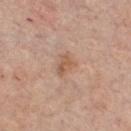| feature | finding |
|---|---|
| workup | catalogued during a skin exam; not biopsied |
| body site | the chest |
| automated metrics | a border-irregularity index near 3/10, internal color variation of about 2 on a 0–10 scale, and peripheral color asymmetry of about 1 |
| acquisition | ~15 mm crop, total-body skin-cancer survey |
| size | ≈2.5 mm |
| patient | male, aged 58–62 |
| illumination | white-light illumination |